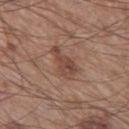A close-up tile cropped from a whole-body skin photograph, about 15 mm across. The subject is a male roughly 60 years of age. The lesion is on the leg. Imaged with white-light lighting.